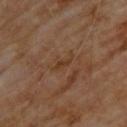Q: What is the anatomic site?
A: the upper back
Q: Who is the patient?
A: male, aged approximately 70
Q: Lesion size?
A: about 3 mm
Q: How was this image acquired?
A: ~15 mm tile from a whole-body skin photo